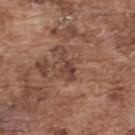Clinical impression: Imaged during a routine full-body skin examination; the lesion was not biopsied and no histopathology is available. Image and clinical context: The total-body-photography lesion software estimated an area of roughly 4 mm² and a shape eccentricity near 0.9. And it measured border irregularity of about 6 on a 0–10 scale, a color-variation rating of about 1/10, and peripheral color asymmetry of about 0.5. The analysis additionally found an automated nevus-likeness rating near 0 out of 100 and a lesion-detection confidence of about 85/100. A male subject, aged around 75. Cropped from a whole-body photographic skin survey; the tile spans about 15 mm. About 3.5 mm across. The tile uses white-light illumination. From the upper back.